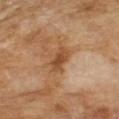This lesion was catalogued during total-body skin photography and was not selected for biopsy.
Captured under cross-polarized illumination.
A 15 mm close-up tile from a total-body photography series done for melanoma screening.
A male subject in their mid- to late 60s.
An algorithmic analysis of the crop reported an area of roughly 5 mm² and two-axis asymmetry of about 0.25.